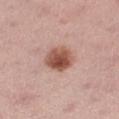follow-up: total-body-photography surveillance lesion; no biopsy | lesion size: ≈3.5 mm | site: the right thigh | acquisition: ~15 mm crop, total-body skin-cancer survey | automated metrics: a footprint of about 9 mm², an eccentricity of roughly 0.55, and a shape-asymmetry score of about 0.15 (0 = symmetric); an average lesion color of about L≈52 a*≈24 b*≈28 (CIELAB), about 15 CIELAB-L* units darker than the surrounding skin, and a lesion-to-skin contrast of about 10.5 (normalized; higher = more distinct) | patient: female, aged 33 to 37 | tile lighting: white-light.